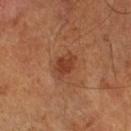* notes · total-body-photography surveillance lesion; no biopsy
* illumination · cross-polarized illumination
* acquisition · total-body-photography crop, ~15 mm field of view
* patient · male, approximately 65 years of age
* automated metrics · a footprint of about 5.5 mm² and a shape-asymmetry score of about 0.2 (0 = symmetric); a normalized border contrast of about 7.5; an automated nevus-likeness rating near 80 out of 100 and a detector confidence of about 100 out of 100 that the crop contains a lesion
* size · about 3 mm